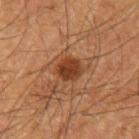Recorded during total-body skin imaging; not selected for excision or biopsy. Longest diameter approximately 3 mm. On the arm. A 15 mm close-up tile from a total-body photography series done for melanoma screening. The subject is a male about 65 years old. Imaged with cross-polarized lighting.